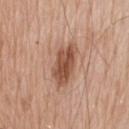Findings:
- acquisition — total-body-photography crop, ~15 mm field of view
- lighting — white-light illumination
- patient — male, roughly 80 years of age
- location — the head or neck
- lesion size — ~5 mm (longest diameter)